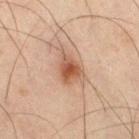biopsy status = no biopsy performed (imaged during a skin exam); location = the right thigh; patient = male, about 45 years old; image = 15 mm crop, total-body photography.